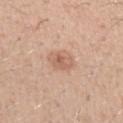This lesion was catalogued during total-body skin photography and was not selected for biopsy.
Imaged with white-light lighting.
From the arm.
Cropped from a total-body skin-imaging series; the visible field is about 15 mm.
A male patient, aged 28–32.
Approximately 2.5 mm at its widest.